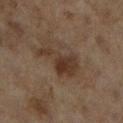Q: Was a biopsy performed?
A: imaged on a skin check; not biopsied
Q: What are the patient's age and sex?
A: female, in their 60s
Q: Lesion location?
A: the right lower leg
Q: How was this image acquired?
A: ~15 mm tile from a whole-body skin photo
Q: What did automated image analysis measure?
A: a lesion area of about 11 mm² and a shape-asymmetry score of about 0.5 (0 = symmetric); a lesion color around L≈30 a*≈14 b*≈23 in CIELAB, roughly 8 lightness units darker than nearby skin, and a lesion-to-skin contrast of about 8 (normalized; higher = more distinct); a classifier nevus-likeness of about 20/100 and a detector confidence of about 100 out of 100 that the crop contains a lesion
Q: Illumination type?
A: cross-polarized